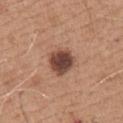No biopsy was performed on this lesion — it was imaged during a full skin examination and was not determined to be concerning. This image is a 15 mm lesion crop taken from a total-body photograph. A male subject aged 63 to 67. This is a white-light tile. Longest diameter approximately 3.5 mm. The lesion is located on the chest.